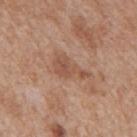follow-up: total-body-photography surveillance lesion; no biopsy
imaging modality: total-body-photography crop, ~15 mm field of view
TBP lesion metrics: an average lesion color of about L≈52 a*≈20 b*≈30 (CIELAB) and roughly 8 lightness units darker than nearby skin; a within-lesion color-variation index near 2.5/10 and peripheral color asymmetry of about 1; a nevus-likeness score of about 0/100 and lesion-presence confidence of about 100/100
subject: male, about 65 years old
illumination: white-light illumination
anatomic site: the mid back